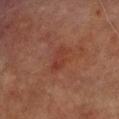| field | value |
|---|---|
| image source | ~15 mm crop, total-body skin-cancer survey |
| patient | male, about 70 years old |
| illumination | cross-polarized illumination |
| diameter | ≈3.5 mm |
| body site | the right lower leg |
| TBP lesion metrics | an average lesion color of about L≈30 a*≈22 b*≈24 (CIELAB), about 5 CIELAB-L* units darker than the surrounding skin, and a lesion-to-skin contrast of about 5 (normalized; higher = more distinct); a border-irregularity index near 3/10, a within-lesion color-variation index near 1.5/10, and radial color variation of about 0.5; a classifier nevus-likeness of about 0/100 and lesion-presence confidence of about 100/100 |